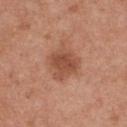{
  "biopsy_status": "not biopsied; imaged during a skin examination",
  "site": "chest",
  "patient": {
    "sex": "female",
    "age_approx": 30
  },
  "lesion_size": {
    "long_diameter_mm_approx": 3.5
  },
  "automated_metrics": {
    "cielab_L": 50,
    "cielab_a": 24,
    "cielab_b": 32,
    "vs_skin_darker_L": 10.0,
    "vs_skin_contrast_norm": 7.5,
    "color_variation_0_10": 2.5,
    "peripheral_color_asymmetry": 1.0,
    "nevus_likeness_0_100": 30,
    "lesion_detection_confidence_0_100": 100
  },
  "lighting": "white-light",
  "image": {
    "source": "total-body photography crop",
    "field_of_view_mm": 15
  }
}